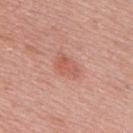Recorded during total-body skin imaging; not selected for excision or biopsy. The recorded lesion diameter is about 3.5 mm. A male patient approximately 50 years of age. An algorithmic analysis of the crop reported a mean CIELAB color near L≈58 a*≈26 b*≈29, about 7 CIELAB-L* units darker than the surrounding skin, and a lesion-to-skin contrast of about 5 (normalized; higher = more distinct). It also reported a border-irregularity rating of about 3/10 and a color-variation rating of about 2/10. And it measured a classifier nevus-likeness of about 55/100. A lesion tile, about 15 mm wide, cut from a 3D total-body photograph. Imaged with white-light lighting. The lesion is on the upper back.